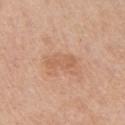| feature | finding |
|---|---|
| location | the left upper arm |
| acquisition | total-body-photography crop, ~15 mm field of view |
| lesion size | about 3.5 mm |
| image-analysis metrics | a footprint of about 4.5 mm², an outline eccentricity of about 0.95 (0 = round, 1 = elongated), and a shape-asymmetry score of about 0.4 (0 = symmetric); an average lesion color of about L≈60 a*≈22 b*≈34 (CIELAB), about 7 CIELAB-L* units darker than the surrounding skin, and a normalized border contrast of about 5 |
| illumination | white-light |
| subject | female, aged approximately 65 |